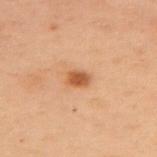workup = catalogued during a skin exam; not biopsied
diameter = ~2.5 mm (longest diameter)
body site = the upper back
image source = ~15 mm crop, total-body skin-cancer survey
subject = female, about 55 years old